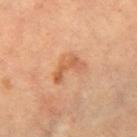- biopsy status — no biopsy performed (imaged during a skin exam)
- lesion diameter — ≈4 mm
- location — the leg
- imaging modality — ~15 mm crop, total-body skin-cancer survey
- patient — roughly 60 years of age
- lighting — cross-polarized illumination